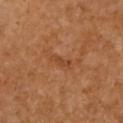Notes:
• workup — imaged on a skin check; not biopsied
• image source — ~15 mm crop, total-body skin-cancer survey
• patient — female, aged approximately 55
• automated metrics — a lesion area of about 2 mm² and an outline eccentricity of about 0.95 (0 = round, 1 = elongated); border irregularity of about 5 on a 0–10 scale, a within-lesion color-variation index near 0/10, and peripheral color asymmetry of about 0
• lesion diameter — about 2.5 mm
• location — the chest